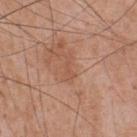  biopsy_status: not biopsied; imaged during a skin examination
  patient:
    sex: male
    age_approx: 55
  lighting: white-light
  site: chest
  lesion_size:
    long_diameter_mm_approx: 3.0
  image:
    source: total-body photography crop
    field_of_view_mm: 15